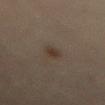{
  "image": {
    "source": "total-body photography crop",
    "field_of_view_mm": 15
  },
  "lesion_size": {
    "long_diameter_mm_approx": 3.0
  },
  "patient": {
    "sex": "female",
    "age_approx": 60
  },
  "lighting": "cross-polarized",
  "site": "lower back"
}